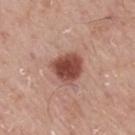Impression: Part of a total-body skin-imaging series; this lesion was reviewed on a skin check and was not flagged for biopsy. Acquisition and patient details: The subject is a male about 75 years old. The lesion is located on the mid back. Imaged with white-light lighting. Cropped from a total-body skin-imaging series; the visible field is about 15 mm. The recorded lesion diameter is about 4 mm.